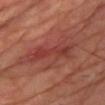biopsy status: catalogued during a skin exam; not biopsied | TBP lesion metrics: a footprint of about 12 mm², a shape eccentricity near 0.9, and a symmetry-axis asymmetry near 0.4 | lesion diameter: ≈6 mm | patient: male, approximately 65 years of age | image source: 15 mm crop, total-body photography | tile lighting: cross-polarized | anatomic site: the chest.